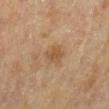Captured during whole-body skin photography for melanoma surveillance; the lesion was not biopsied. A female subject in their mid- to late 50s. Cropped from a total-body skin-imaging series; the visible field is about 15 mm. The lesion is on the left lower leg.